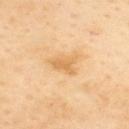Case summary:
* workup · no biopsy performed (imaged during a skin exam)
* imaging modality · ~15 mm crop, total-body skin-cancer survey
* subject · male, approximately 55 years of age
* automated lesion analysis · a lesion area of about 4.5 mm², an outline eccentricity of about 0.7 (0 = round, 1 = elongated), and two-axis asymmetry of about 0.35; an average lesion color of about L≈71 a*≈21 b*≈47 (CIELAB), a lesion–skin lightness drop of about 9, and a normalized lesion–skin contrast near 6; a border-irregularity index near 4/10, a within-lesion color-variation index near 1.5/10, and peripheral color asymmetry of about 0.5
* diameter · about 3 mm
* location · the upper back